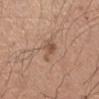Assessment:
Recorded during total-body skin imaging; not selected for excision or biopsy.
Context:
About 2.5 mm across. This is a white-light tile. A male patient, in their mid-40s. The lesion is located on the left forearm. A region of skin cropped from a whole-body photographic capture, roughly 15 mm wide.